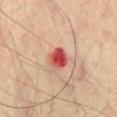| field | value |
|---|---|
| biopsy status | no biopsy performed (imaged during a skin exam) |
| patient | male, roughly 75 years of age |
| location | the front of the torso |
| image-analysis metrics | a border-irregularity rating of about 1.5/10, a within-lesion color-variation index near 5.5/10, and peripheral color asymmetry of about 2; an automated nevus-likeness rating near 0 out of 100 and lesion-presence confidence of about 100/100 |
| lesion diameter | about 2.5 mm |
| illumination | cross-polarized illumination |
| acquisition | total-body-photography crop, ~15 mm field of view |